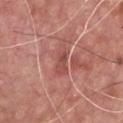Q: Was this lesion biopsied?
A: imaged on a skin check; not biopsied
Q: How was this image acquired?
A: 15 mm crop, total-body photography
Q: Where on the body is the lesion?
A: the chest
Q: Illumination type?
A: white-light
Q: Automated lesion metrics?
A: an average lesion color of about L≈50 a*≈27 b*≈26 (CIELAB), a lesion–skin lightness drop of about 9, and a lesion-to-skin contrast of about 6 (normalized; higher = more distinct); a nevus-likeness score of about 0/100 and a lesion-detection confidence of about 55/100
Q: Who is the patient?
A: male, approximately 60 years of age